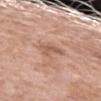Clinical impression:
No biopsy was performed on this lesion — it was imaged during a full skin examination and was not determined to be concerning.
Acquisition and patient details:
A lesion tile, about 15 mm wide, cut from a 3D total-body photograph. A female subject, aged 68–72. The lesion is located on the left upper arm. About 2.5 mm across. The tile uses white-light illumination. The total-body-photography lesion software estimated a footprint of about 4.5 mm², an eccentricity of roughly 0.6, and a symmetry-axis asymmetry near 0.35. The analysis additionally found roughly 9 lightness units darker than nearby skin and a normalized border contrast of about 6. The analysis additionally found border irregularity of about 4 on a 0–10 scale and a color-variation rating of about 1.5/10. The software also gave a detector confidence of about 100 out of 100 that the crop contains a lesion.